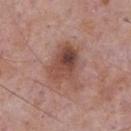The lesion was tiled from a total-body skin photograph and was not biopsied. The lesion's longest dimension is about 5.5 mm. The lesion-visualizer software estimated an average lesion color of about L≈47 a*≈22 b*≈25 (CIELAB), roughly 12 lightness units darker than nearby skin, and a normalized lesion–skin contrast near 8.5. And it measured a border-irregularity rating of about 4/10, a within-lesion color-variation index near 9/10, and peripheral color asymmetry of about 3.5. The patient is a male aged around 75. From the front of the torso. Captured under white-light illumination. This image is a 15 mm lesion crop taken from a total-body photograph.